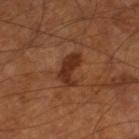No biopsy was performed on this lesion — it was imaged during a full skin examination and was not determined to be concerning. The lesion is located on the left leg. A male patient aged approximately 65. A 15 mm close-up tile from a total-body photography series done for melanoma screening.